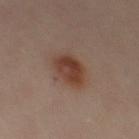The lesion was tiled from a total-body skin photograph and was not biopsied. The lesion is on the left thigh. A 15 mm close-up tile from a total-body photography series done for melanoma screening. This is a cross-polarized tile. Longest diameter approximately 4 mm. The subject is a female aged 38–42.